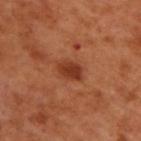Q: Is there a histopathology result?
A: total-body-photography surveillance lesion; no biopsy
Q: What are the patient's age and sex?
A: male, approximately 50 years of age
Q: What is the anatomic site?
A: the upper back
Q: What is the imaging modality?
A: 15 mm crop, total-body photography